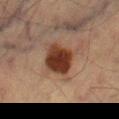{
  "biopsy_status": "not biopsied; imaged during a skin examination"
}